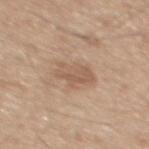Captured during whole-body skin photography for melanoma surveillance; the lesion was not biopsied. An algorithmic analysis of the crop reported a lesion color around L≈57 a*≈17 b*≈30 in CIELAB and roughly 8 lightness units darker than nearby skin. It also reported a border-irregularity rating of about 4/10 and a color-variation rating of about 3/10. The analysis additionally found a detector confidence of about 100 out of 100 that the crop contains a lesion. A male patient, in their mid- to late 40s. Located on the back. Imaged with white-light lighting. The lesion's longest dimension is about 4 mm. This image is a 15 mm lesion crop taken from a total-body photograph.